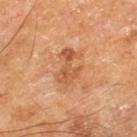This lesion was catalogued during total-body skin photography and was not selected for biopsy. On the leg. Captured under cross-polarized illumination. The total-body-photography lesion software estimated a within-lesion color-variation index near 4.5/10 and a peripheral color-asymmetry measure near 1.5. A lesion tile, about 15 mm wide, cut from a 3D total-body photograph. A male subject in their mid-60s. The lesion's longest dimension is about 4.5 mm.